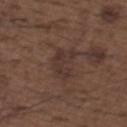notes: no biopsy performed (imaged during a skin exam)
illumination: white-light illumination
automated lesion analysis: a shape eccentricity near 0.5; an average lesion color of about L≈33 a*≈16 b*≈21 (CIELAB), a lesion–skin lightness drop of about 5, and a normalized lesion–skin contrast near 5.5; a border-irregularity index near 5/10 and radial color variation of about 1; lesion-presence confidence of about 95/100
anatomic site: the upper back
subject: male, aged approximately 50
imaging modality: total-body-photography crop, ~15 mm field of view
diameter: ≈3.5 mm